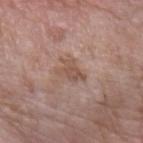Captured during whole-body skin photography for melanoma surveillance; the lesion was not biopsied. A female subject aged around 75. About 3 mm across. Imaged with white-light lighting. The lesion is on the arm. A region of skin cropped from a whole-body photographic capture, roughly 15 mm wide.